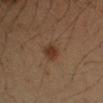Q: Is there a histopathology result?
A: no biopsy performed (imaged during a skin exam)
Q: Patient demographics?
A: male, about 50 years old
Q: What is the imaging modality?
A: ~15 mm crop, total-body skin-cancer survey
Q: What is the lesion's diameter?
A: about 2.5 mm
Q: What is the anatomic site?
A: the left upper arm
Q: How was the tile lit?
A: cross-polarized